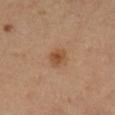Clinical impression:
The lesion was tiled from a total-body skin photograph and was not biopsied.
Background:
Imaged with cross-polarized lighting. From the right lower leg. The recorded lesion diameter is about 2.5 mm. A 15 mm close-up tile from a total-body photography series done for melanoma screening. A female subject in their mid-50s.